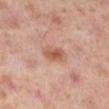Impression:
Recorded during total-body skin imaging; not selected for excision or biopsy.
Context:
The subject is a female approximately 40 years of age. On the left lower leg. Cropped from a total-body skin-imaging series; the visible field is about 15 mm.